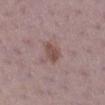{"biopsy_status": "not biopsied; imaged during a skin examination", "image": {"source": "total-body photography crop", "field_of_view_mm": 15}, "lesion_size": {"long_diameter_mm_approx": 2.5}, "lighting": "white-light", "site": "right lower leg", "patient": {"sex": "female", "age_approx": 40}}